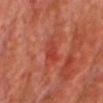| field | value |
|---|---|
| subject | male, approximately 60 years of age |
| acquisition | ~15 mm crop, total-body skin-cancer survey |
| automated lesion analysis | border irregularity of about 3.5 on a 0–10 scale and a within-lesion color-variation index near 2.5/10; a classifier nevus-likeness of about 0/100 and a lesion-detection confidence of about 95/100 |
| site | the front of the torso |
| illumination | cross-polarized |